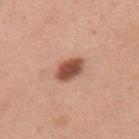biopsy status: total-body-photography surveillance lesion; no biopsy | size: ~3.5 mm (longest diameter) | anatomic site: the back | patient: female, in their 50s | tile lighting: white-light | imaging modality: ~15 mm tile from a whole-body skin photo.